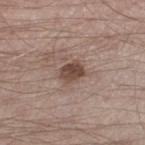{
  "biopsy_status": "not biopsied; imaged during a skin examination",
  "image": {
    "source": "total-body photography crop",
    "field_of_view_mm": 15
  },
  "patient": {
    "sex": "male",
    "age_approx": 35
  },
  "lesion_size": {
    "long_diameter_mm_approx": 3.0
  },
  "site": "leg"
}